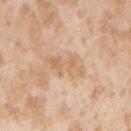The lesion was photographed on a routine skin check and not biopsied; there is no pathology result. The tile uses white-light illumination. A female subject, aged approximately 25. On the arm. A 15 mm close-up extracted from a 3D total-body photography capture. The lesion's longest dimension is about 5 mm.